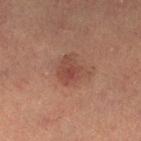The lesion was tiled from a total-body skin photograph and was not biopsied. The patient is a female approximately 60 years of age. Located on the left lower leg. Automated image analysis of the tile measured an eccentricity of roughly 0.25 and a symmetry-axis asymmetry near 0.25. It also reported a color-variation rating of about 4/10 and peripheral color asymmetry of about 1.5. It also reported a nevus-likeness score of about 55/100 and a lesion-detection confidence of about 100/100. A close-up tile cropped from a whole-body skin photograph, about 15 mm across. Imaged with cross-polarized lighting.